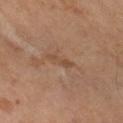biopsy status = catalogued during a skin exam; not biopsied
diameter = ~3 mm (longest diameter)
acquisition = 15 mm crop, total-body photography
patient = male, in their mid-60s
tile lighting = cross-polarized illumination
automated metrics = border irregularity of about 4.5 on a 0–10 scale and internal color variation of about 0 on a 0–10 scale
body site = the left forearm